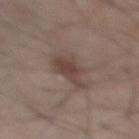Impression:
This lesion was catalogued during total-body skin photography and was not selected for biopsy.
Acquisition and patient details:
Automated image analysis of the tile measured an area of roughly 9 mm² and two-axis asymmetry of about 0.4. The software also gave lesion-presence confidence of about 100/100. A male patient roughly 55 years of age. A 15 mm close-up tile from a total-body photography series done for melanoma screening. This is a white-light tile. From the right lower leg.